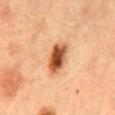<case>
<biopsy_status>not biopsied; imaged during a skin examination</biopsy_status>
<image>
  <source>total-body photography crop</source>
  <field_of_view_mm>15</field_of_view_mm>
</image>
<patient>
  <sex>female</sex>
  <age_approx>60</age_approx>
</patient>
<site>abdomen</site>
</case>